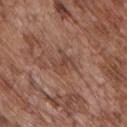Recorded during total-body skin imaging; not selected for excision or biopsy.
From the chest.
Approximately 3 mm at its widest.
Automated tile analysis of the lesion measured border irregularity of about 4.5 on a 0–10 scale, a within-lesion color-variation index near 4/10, and a peripheral color-asymmetry measure near 1.5. It also reported an automated nevus-likeness rating near 0 out of 100 and a detector confidence of about 100 out of 100 that the crop contains a lesion.
This is a white-light tile.
A lesion tile, about 15 mm wide, cut from a 3D total-body photograph.
The patient is a male roughly 70 years of age.